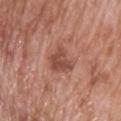biopsy_status: not biopsied; imaged during a skin examination
lesion_size:
  long_diameter_mm_approx: 4.0
lighting: white-light
patient:
  sex: male
  age_approx: 70
image:
  source: total-body photography crop
  field_of_view_mm: 15
site: back
automated_metrics:
  cielab_L: 49
  cielab_a: 24
  cielab_b: 28
  vs_skin_darker_L: 10.0
  color_variation_0_10: 4.0
  peripheral_color_asymmetry: 1.5
  nevus_likeness_0_100: 20
  lesion_detection_confidence_0_100: 100